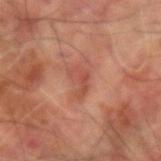patient = male, aged 58 to 62; tile lighting = cross-polarized; location = the arm; lesion size = about 3.5 mm; image source = 15 mm crop, total-body photography.